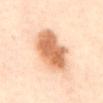An algorithmic analysis of the crop reported a shape eccentricity near 0.65 and two-axis asymmetry of about 0.15. From the abdomen. The tile uses cross-polarized illumination. A 15 mm close-up extracted from a 3D total-body photography capture. A female patient in their 40s.